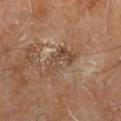Part of a total-body skin-imaging series; this lesion was reviewed on a skin check and was not flagged for biopsy. The lesion is on the right leg. Cropped from a total-body skin-imaging series; the visible field is about 15 mm. A male subject in their 60s.